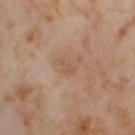Recorded during total-body skin imaging; not selected for excision or biopsy. A 15 mm crop from a total-body photograph taken for skin-cancer surveillance. The lesion is located on the right thigh. A female patient aged around 55.